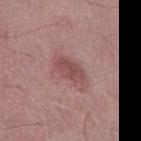A 15 mm crop from a total-body photograph taken for skin-cancer surveillance. The recorded lesion diameter is about 3.5 mm. Captured under white-light illumination. Automated image analysis of the tile measured a mean CIELAB color near L≈48 a*≈24 b*≈20, about 10 CIELAB-L* units darker than the surrounding skin, and a normalized lesion–skin contrast near 7. The software also gave an automated nevus-likeness rating near 30 out of 100 and a lesion-detection confidence of about 100/100. The lesion is located on the left thigh. The subject is a male aged 53 to 57.